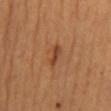Imaged during a routine full-body skin examination; the lesion was not biopsied and no histopathology is available. From the mid back. A female subject. The lesion's longest dimension is about 3 mm. A 15 mm crop from a total-body photograph taken for skin-cancer surveillance. An algorithmic analysis of the crop reported a footprint of about 3 mm², a shape eccentricity near 0.9, and a symmetry-axis asymmetry near 0.3. It also reported an average lesion color of about L≈43 a*≈24 b*≈35 (CIELAB) and a normalized border contrast of about 7.5. The software also gave a border-irregularity index near 3.5/10, internal color variation of about 0 on a 0–10 scale, and radial color variation of about 0. It also reported a classifier nevus-likeness of about 50/100. This is a cross-polarized tile.